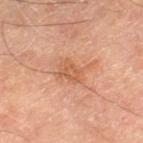Q: How was this image acquired?
A: total-body-photography crop, ~15 mm field of view
Q: How was the tile lit?
A: cross-polarized illumination
Q: Where on the body is the lesion?
A: the left thigh
Q: Who is the patient?
A: male, about 70 years old
Q: How large is the lesion?
A: about 4 mm